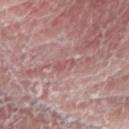biopsy_status: not biopsied; imaged during a skin examination
lighting: white-light
automated_metrics:
  nevus_likeness_0_100: 0
  lesion_detection_confidence_0_100: 55
image:
  source: total-body photography crop
  field_of_view_mm: 15
site: right forearm
lesion_size:
  long_diameter_mm_approx: 3.0
patient:
  sex: male
  age_approx: 60